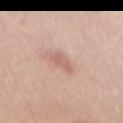| field | value |
|---|---|
| follow-up | total-body-photography surveillance lesion; no biopsy |
| location | the chest |
| acquisition | ~15 mm tile from a whole-body skin photo |
| subject | female, aged around 45 |
| size | ~3.5 mm (longest diameter) |